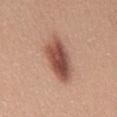<tbp_lesion>
<biopsy_status>not biopsied; imaged during a skin examination</biopsy_status>
<automated_metrics>
  <shape_asymmetry>0.15</shape_asymmetry>
  <border_irregularity_0_10>2.0</border_irregularity_0_10>
  <color_variation_0_10>7.5</color_variation_0_10>
  <peripheral_color_asymmetry>2.5</peripheral_color_asymmetry>
  <lesion_detection_confidence_0_100>100</lesion_detection_confidence_0_100>
</automated_metrics>
<image>
  <source>total-body photography crop</source>
  <field_of_view_mm>15</field_of_view_mm>
</image>
<patient>
  <sex>female</sex>
  <age_approx>35</age_approx>
</patient>
<lesion_size>
  <long_diameter_mm_approx>6.5</long_diameter_mm_approx>
</lesion_size>
<site>back</site>
</tbp_lesion>